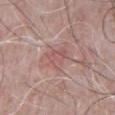workup: total-body-photography surveillance lesion; no biopsy
diameter: ≈3 mm
subject: male, aged around 65
lighting: white-light
image-analysis metrics: a lesion area of about 6 mm², an outline eccentricity of about 0.55 (0 = round, 1 = elongated), and a symmetry-axis asymmetry near 0.4; an average lesion color of about L≈55 a*≈23 b*≈21 (CIELAB), about 7 CIELAB-L* units darker than the surrounding skin, and a lesion-to-skin contrast of about 5 (normalized; higher = more distinct); a nevus-likeness score of about 0/100 and lesion-presence confidence of about 100/100
image: ~15 mm tile from a whole-body skin photo
body site: the chest